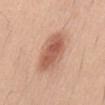Impression:
No biopsy was performed on this lesion — it was imaged during a full skin examination and was not determined to be concerning.
Clinical summary:
An algorithmic analysis of the crop reported about 13 CIELAB-L* units darker than the surrounding skin and a normalized border contrast of about 8.5. It also reported a border-irregularity index near 2/10, a color-variation rating of about 4.5/10, and a peripheral color-asymmetry measure near 1.5. And it measured an automated nevus-likeness rating near 95 out of 100 and a lesion-detection confidence of about 100/100. The subject is a male in their 40s. From the abdomen. A 15 mm crop from a total-body photograph taken for skin-cancer surveillance. This is a white-light tile.